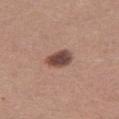image = total-body-photography crop, ~15 mm field of view | lighting = white-light | patient = female, aged approximately 35 | site = the right thigh | size = ≈3 mm | image-analysis metrics = an average lesion color of about L≈45 a*≈20 b*≈23 (CIELAB) and a normalized lesion–skin contrast near 11.5.